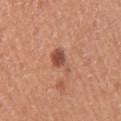This is a white-light tile. On the right upper arm. The lesion-visualizer software estimated a border-irregularity rating of about 4/10, a within-lesion color-variation index near 3.5/10, and radial color variation of about 1. And it measured a classifier nevus-likeness of about 80/100 and a lesion-detection confidence of about 100/100. A 15 mm close-up extracted from a 3D total-body photography capture. A female subject in their 60s. About 3.5 mm across.